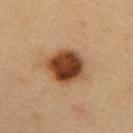No biopsy was performed on this lesion — it was imaged during a full skin examination and was not determined to be concerning.
Cropped from a whole-body photographic skin survey; the tile spans about 15 mm.
The patient is a male aged around 50.
About 4 mm across.
The lesion is on the abdomen.
Captured under cross-polarized illumination.